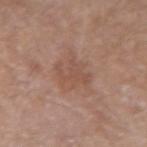follow-up: total-body-photography surveillance lesion; no biopsy | image-analysis metrics: a shape eccentricity near 0.5 and two-axis asymmetry of about 0.3; border irregularity of about 3.5 on a 0–10 scale and peripheral color asymmetry of about 1; an automated nevus-likeness rating near 0 out of 100 | size: ≈4.5 mm | illumination: white-light | anatomic site: the left forearm | subject: male, aged 68–72 | image: ~15 mm crop, total-body skin-cancer survey.